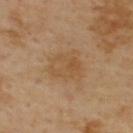Case summary:
* biopsy status: imaged on a skin check; not biopsied
* automated lesion analysis: an eccentricity of roughly 0.65; a classifier nevus-likeness of about 0/100 and a lesion-detection confidence of about 100/100
* image: ~15 mm tile from a whole-body skin photo
* lesion diameter: ~4 mm (longest diameter)
* site: the upper back
* patient: male, in their mid-50s
* illumination: cross-polarized